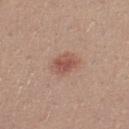<case>
  <biopsy_status>not biopsied; imaged during a skin examination</biopsy_status>
  <site>left thigh</site>
  <lighting>white-light</lighting>
  <patient>
    <sex>female</sex>
    <age_approx>25</age_approx>
  </patient>
  <lesion_size>
    <long_diameter_mm_approx>3.5</long_diameter_mm_approx>
  </lesion_size>
  <image>
    <source>total-body photography crop</source>
    <field_of_view_mm>15</field_of_view_mm>
  </image>
</case>